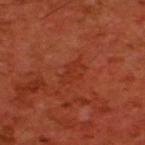This lesion was catalogued during total-body skin photography and was not selected for biopsy.
Automated tile analysis of the lesion measured a lesion color around L≈32 a*≈31 b*≈34 in CIELAB, roughly 4 lightness units darker than nearby skin, and a normalized border contrast of about 4.5. And it measured a classifier nevus-likeness of about 0/100 and lesion-presence confidence of about 100/100.
Located on the upper back.
A male subject approximately 60 years of age.
This image is a 15 mm lesion crop taken from a total-body photograph.